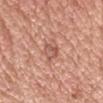Q: Was this lesion biopsied?
A: imaged on a skin check; not biopsied
Q: How large is the lesion?
A: about 3 mm
Q: How was this image acquired?
A: ~15 mm crop, total-body skin-cancer survey
Q: Who is the patient?
A: male, aged 53–57
Q: What lighting was used for the tile?
A: white-light
Q: Lesion location?
A: the head or neck